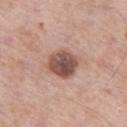Impression: Part of a total-body skin-imaging series; this lesion was reviewed on a skin check and was not flagged for biopsy. Acquisition and patient details: A close-up tile cropped from a whole-body skin photograph, about 15 mm across. The patient is a male about 55 years old. Located on the left thigh. The lesion's longest dimension is about 3.5 mm. The tile uses white-light illumination.